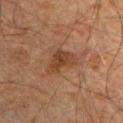notes: imaged on a skin check; not biopsied
lesion size: about 4 mm
patient: male, aged approximately 60
acquisition: 15 mm crop, total-body photography
anatomic site: the front of the torso